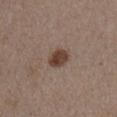Clinical impression:
Part of a total-body skin-imaging series; this lesion was reviewed on a skin check and was not flagged for biopsy.
Clinical summary:
An algorithmic analysis of the crop reported a lesion area of about 5.5 mm². It also reported an average lesion color of about L≈41 a*≈17 b*≈24 (CIELAB) and about 12 CIELAB-L* units darker than the surrounding skin. The analysis additionally found a border-irregularity index near 1.5/10, a within-lesion color-variation index near 3.5/10, and a peripheral color-asymmetry measure near 1. And it measured an automated nevus-likeness rating near 95 out of 100. About 3 mm across. From the right upper arm. A male subject approximately 55 years of age. A roughly 15 mm field-of-view crop from a total-body skin photograph. Captured under white-light illumination.